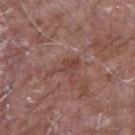notes: no biopsy performed (imaged during a skin exam)
subject: male, aged around 65
TBP lesion metrics: a lesion area of about 4 mm², an eccentricity of roughly 0.9, and two-axis asymmetry of about 0.45; a peripheral color-asymmetry measure near 1
lesion diameter: ~4 mm (longest diameter)
image: 15 mm crop, total-body photography
anatomic site: the right upper arm